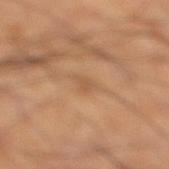Imaged during a routine full-body skin examination; the lesion was not biopsied and no histopathology is available.
Located on the right lower leg.
A roughly 15 mm field-of-view crop from a total-body skin photograph.
A male patient, aged approximately 60.
The tile uses cross-polarized illumination.
Approximately 2.5 mm at its widest.
The lesion-visualizer software estimated a footprint of about 3 mm², an eccentricity of roughly 0.75, and two-axis asymmetry of about 0.35. It also reported a nevus-likeness score of about 0/100 and lesion-presence confidence of about 100/100.